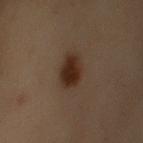| feature | finding |
|---|---|
| notes | total-body-photography surveillance lesion; no biopsy |
| subject | female, aged around 50 |
| location | the left upper arm |
| image source | ~15 mm tile from a whole-body skin photo |